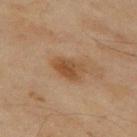Impression:
No biopsy was performed on this lesion — it was imaged during a full skin examination and was not determined to be concerning.
Acquisition and patient details:
This image is a 15 mm lesion crop taken from a total-body photograph. On the left thigh. The subject is a female roughly 60 years of age.